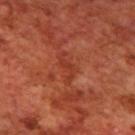Clinical impression: Imaged during a routine full-body skin examination; the lesion was not biopsied and no histopathology is available. Background: Automated image analysis of the tile measured roughly 6 lightness units darker than nearby skin and a normalized lesion–skin contrast near 5.5. On the upper back. A male patient in their 70s. The lesion's longest dimension is about 4 mm. A 15 mm crop from a total-body photograph taken for skin-cancer surveillance. Imaged with cross-polarized lighting.